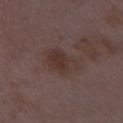Recorded during total-body skin imaging; not selected for excision or biopsy. Automated tile analysis of the lesion measured a footprint of about 11 mm², an eccentricity of roughly 0.75, and a shape-asymmetry score of about 0.25 (0 = symmetric). The analysis additionally found a peripheral color-asymmetry measure near 1. The software also gave an automated nevus-likeness rating near 10 out of 100 and a lesion-detection confidence of about 100/100. The subject is a female aged around 35. Cropped from a total-body skin-imaging series; the visible field is about 15 mm. From the right thigh. Measured at roughly 5 mm in maximum diameter. Captured under white-light illumination.